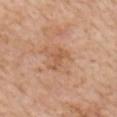<tbp_lesion>
  <biopsy_status>not biopsied; imaged during a skin examination</biopsy_status>
  <site>chest</site>
  <patient>
    <sex>female</sex>
    <age_approx>70</age_approx>
  </patient>
  <automated_metrics>
    <cielab_L>56</cielab_L>
    <cielab_a>22</cielab_a>
    <cielab_b>35</cielab_b>
    <vs_skin_darker_L>7.0</vs_skin_darker_L>
    <vs_skin_contrast_norm>5.5</vs_skin_contrast_norm>
    <border_irregularity_0_10>5.5</border_irregularity_0_10>
    <color_variation_0_10>1.0</color_variation_0_10>
    <peripheral_color_asymmetry>0.5</peripheral_color_asymmetry>
    <nevus_likeness_0_100>0</nevus_likeness_0_100>
    <lesion_detection_confidence_0_100>100</lesion_detection_confidence_0_100>
  </automated_metrics>
  <image>
    <source>total-body photography crop</source>
    <field_of_view_mm>15</field_of_view_mm>
  </image>
  <lighting>white-light</lighting>
</tbp_lesion>